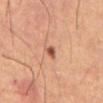notes: catalogued during a skin exam; not biopsied | lesion size: about 1.5 mm | imaging modality: ~15 mm crop, total-body skin-cancer survey | subject: male, in their mid- to late 50s | site: the abdomen | image-analysis metrics: a lesion area of about 2 mm², an outline eccentricity of about 0.45 (0 = round, 1 = elongated), and a shape-asymmetry score of about 0.3 (0 = symmetric); a border-irregularity rating of about 2.5/10, internal color variation of about 2 on a 0–10 scale, and a peripheral color-asymmetry measure near 1; a nevus-likeness score of about 85/100 and a lesion-detection confidence of about 100/100.